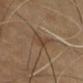The lesion was photographed on a routine skin check and not biopsied; there is no pathology result. Cropped from a whole-body photographic skin survey; the tile spans about 15 mm. Located on the right thigh. The patient is aged approximately 65.